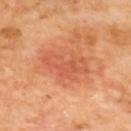| feature | finding |
|---|---|
| workup | imaged on a skin check; not biopsied |
| location | the upper back |
| imaging modality | ~15 mm crop, total-body skin-cancer survey |